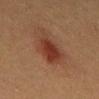Case summary:
* follow-up · total-body-photography surveillance lesion; no biopsy
* patient · male, aged 38–42
* anatomic site · the abdomen
* image source · ~15 mm crop, total-body skin-cancer survey